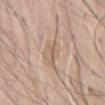Clinical impression: Part of a total-body skin-imaging series; this lesion was reviewed on a skin check and was not flagged for biopsy. Acquisition and patient details: On the front of the torso. A male patient aged approximately 75. A 15 mm close-up extracted from a 3D total-body photography capture.